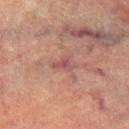The lesion was tiled from a total-body skin photograph and was not biopsied. Approximately 2.5 mm at its widest. Located on the right lower leg. A male patient roughly 75 years of age. An algorithmic analysis of the crop reported an average lesion color of about L≈42 a*≈19 b*≈19 (CIELAB), roughly 7 lightness units darker than nearby skin, and a lesion-to-skin contrast of about 6 (normalized; higher = more distinct). And it measured a nevus-likeness score of about 0/100 and a detector confidence of about 85 out of 100 that the crop contains a lesion. A lesion tile, about 15 mm wide, cut from a 3D total-body photograph.